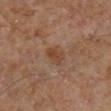Image and clinical context:
This is a cross-polarized tile. Located on the right lower leg. The total-body-photography lesion software estimated a footprint of about 5.5 mm², an eccentricity of roughly 0.75, and a shape-asymmetry score of about 0.2 (0 = symmetric). The analysis additionally found an automated nevus-likeness rating near 0 out of 100 and lesion-presence confidence of about 100/100. The subject is a male aged 58–62. Cropped from a whole-body photographic skin survey; the tile spans about 15 mm.